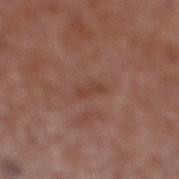Background: Cropped from a total-body skin-imaging series; the visible field is about 15 mm. On the right lower leg. This is a white-light tile. A male subject aged 73 to 77. Automated tile analysis of the lesion measured about 6 CIELAB-L* units darker than the surrounding skin and a normalized border contrast of about 5. The analysis additionally found a classifier nevus-likeness of about 0/100.